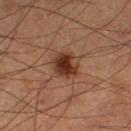Q: Was this lesion biopsied?
A: catalogued during a skin exam; not biopsied
Q: What is the anatomic site?
A: the left lower leg
Q: How was this image acquired?
A: 15 mm crop, total-body photography
Q: How large is the lesion?
A: ≈3 mm
Q: Who is the patient?
A: male, about 60 years old